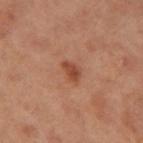No biopsy was performed on this lesion — it was imaged during a full skin examination and was not determined to be concerning.
The lesion is located on the leg.
This image is a 15 mm lesion crop taken from a total-body photograph.
Automated image analysis of the tile measured an area of roughly 3 mm² and two-axis asymmetry of about 0.4. The analysis additionally found a lesion-detection confidence of about 100/100.
A female subject, aged 63–67.
This is a cross-polarized tile.